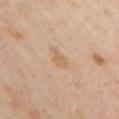Assessment:
The lesion was tiled from a total-body skin photograph and was not biopsied.
Image and clinical context:
This is a cross-polarized tile. Automated tile analysis of the lesion measured a lesion area of about 3.5 mm², an outline eccentricity of about 0.8 (0 = round, 1 = elongated), and a symmetry-axis asymmetry near 0.3. The patient is a female aged approximately 40. From the left arm. The lesion's longest dimension is about 3 mm. A 15 mm crop from a total-body photograph taken for skin-cancer surveillance.